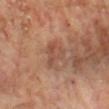workup — no biopsy performed (imaged during a skin exam); patient — male, aged 68 to 72; anatomic site — the chest; imaging modality — ~15 mm crop, total-body skin-cancer survey; lesion diameter — ≈3 mm; lighting — cross-polarized illumination.